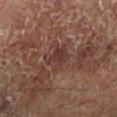follow-up — catalogued during a skin exam; not biopsied | lesion diameter — ~3 mm (longest diameter) | patient — male, aged 63 to 67 | illumination — cross-polarized illumination | body site — the left lower leg | image — ~15 mm tile from a whole-body skin photo.